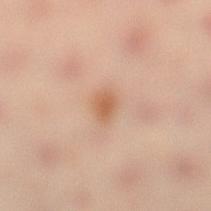The lesion was photographed on a routine skin check and not biopsied; there is no pathology result. The patient is a female roughly 40 years of age. A close-up tile cropped from a whole-body skin photograph, about 15 mm across. Measured at roughly 2.5 mm in maximum diameter. On the leg.